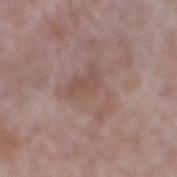{"biopsy_status": "not biopsied; imaged during a skin examination", "site": "right lower leg", "patient": {"sex": "male", "age_approx": 80}, "image": {"source": "total-body photography crop", "field_of_view_mm": 15}}